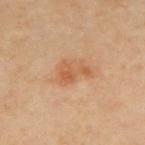Captured during whole-body skin photography for melanoma surveillance; the lesion was not biopsied.